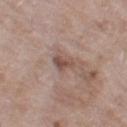Case summary:
* follow-up · catalogued during a skin exam; not biopsied
* body site · the left thigh
* image source · ~15 mm tile from a whole-body skin photo
* tile lighting · white-light
* TBP lesion metrics · a footprint of about 3.5 mm², a shape eccentricity near 0.85, and a symmetry-axis asymmetry near 0.4; a border-irregularity index near 4/10 and internal color variation of about 1.5 on a 0–10 scale; an automated nevus-likeness rating near 0 out of 100 and a lesion-detection confidence of about 85/100
* patient · female, aged approximately 75